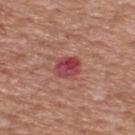Recorded during total-body skin imaging; not selected for excision or biopsy.
A 15 mm close-up extracted from a 3D total-body photography capture.
The tile uses white-light illumination.
The total-body-photography lesion software estimated a border-irregularity rating of about 1.5/10, a color-variation rating of about 7/10, and radial color variation of about 2.5. It also reported an automated nevus-likeness rating near 0 out of 100 and a lesion-detection confidence of about 100/100.
A male subject aged 53–57.
On the back.